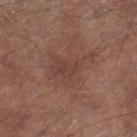Impression:
Recorded during total-body skin imaging; not selected for excision or biopsy.
Image and clinical context:
A 15 mm crop from a total-body photograph taken for skin-cancer surveillance. An algorithmic analysis of the crop reported a mean CIELAB color near L≈42 a*≈20 b*≈23, about 6 CIELAB-L* units darker than the surrounding skin, and a normalized lesion–skin contrast near 5.5. It also reported a border-irregularity index near 7.5/10, a within-lesion color-variation index near 2.5/10, and radial color variation of about 1. From the right lower leg. A male subject, aged 53–57. Imaged with white-light lighting. The lesion's longest dimension is about 5.5 mm.